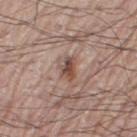Imaged during a routine full-body skin examination; the lesion was not biopsied and no histopathology is available. A region of skin cropped from a whole-body photographic capture, roughly 15 mm wide. A male patient in their 70s. The tile uses white-light illumination. Located on the left leg.